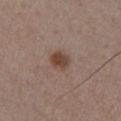biopsy status = no biopsy performed (imaged during a skin exam) | diameter = about 2.5 mm | patient = male, aged 53 to 57 | automated lesion analysis = a lesion color around L≈44 a*≈18 b*≈25 in CIELAB and a normalized border contrast of about 9; a nevus-likeness score of about 95/100 | tile lighting = white-light illumination | location = the front of the torso | image = ~15 mm tile from a whole-body skin photo.